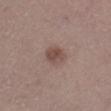| feature | finding |
|---|---|
| body site | the right lower leg |
| subject | female, aged 38–42 |
| diameter | about 3 mm |
| image | total-body-photography crop, ~15 mm field of view |
| image-analysis metrics | a footprint of about 5.5 mm² and an outline eccentricity of about 0.65 (0 = round, 1 = elongated); a within-lesion color-variation index near 3/10 and radial color variation of about 1 |